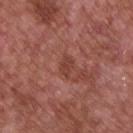Impression: The lesion was photographed on a routine skin check and not biopsied; there is no pathology result. Background: About 2.5 mm across. The patient is a male roughly 65 years of age. The tile uses white-light illumination. The lesion-visualizer software estimated a footprint of about 3.5 mm², a shape eccentricity near 0.8, and a shape-asymmetry score of about 0.4 (0 = symmetric). The analysis additionally found an average lesion color of about L≈42 a*≈26 b*≈27 (CIELAB), about 7 CIELAB-L* units darker than the surrounding skin, and a normalized border contrast of about 6. It also reported a border-irregularity index near 3.5/10 and a within-lesion color-variation index near 2/10. A roughly 15 mm field-of-view crop from a total-body skin photograph. The lesion is on the chest.